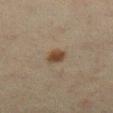The lesion was tiled from a total-body skin photograph and was not biopsied.